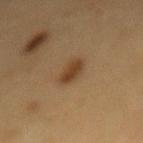Clinical impression:
No biopsy was performed on this lesion — it was imaged during a full skin examination and was not determined to be concerning.
Background:
Automated tile analysis of the lesion measured a lesion area of about 5.5 mm², a shape eccentricity near 0.9, and a shape-asymmetry score of about 0.3 (0 = symmetric). The analysis additionally found a border-irregularity rating of about 3/10 and radial color variation of about 0.5. And it measured a lesion-detection confidence of about 100/100. This image is a 15 mm lesion crop taken from a total-body photograph. This is a cross-polarized tile. The lesion is located on the back. The lesion's longest dimension is about 3.5 mm. A male patient, aged 83 or older.